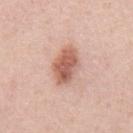<lesion>
<biopsy_status>not biopsied; imaged during a skin examination</biopsy_status>
<lighting>white-light</lighting>
<image>
  <source>total-body photography crop</source>
  <field_of_view_mm>15</field_of_view_mm>
</image>
<automated_metrics>
  <border_irregularity_0_10>1.5</border_irregularity_0_10>
  <color_variation_0_10>5.0</color_variation_0_10>
  <peripheral_color_asymmetry>1.5</peripheral_color_asymmetry>
  <nevus_likeness_0_100>95</nevus_likeness_0_100>
  <lesion_detection_confidence_0_100>100</lesion_detection_confidence_0_100>
</automated_metrics>
<site>mid back</site>
<patient>
  <sex>male</sex>
  <age_approx>55</age_approx>
</patient>
</lesion>